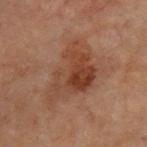This lesion was catalogued during total-body skin photography and was not selected for biopsy. Located on the chest. The lesion-visualizer software estimated a lesion area of about 23 mm² and two-axis asymmetry of about 0.45. The software also gave an average lesion color of about L≈32 a*≈17 b*≈24 (CIELAB) and a normalized border contrast of about 7. And it measured a border-irregularity rating of about 5/10, a color-variation rating of about 5.5/10, and peripheral color asymmetry of about 1.5. The subject is a male roughly 70 years of age. Approximately 7.5 mm at its widest. A 15 mm close-up extracted from a 3D total-body photography capture.